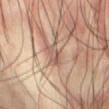This lesion was catalogued during total-body skin photography and was not selected for biopsy. The lesion is located on the abdomen. Imaged with cross-polarized lighting. The subject is a male aged 68–72. Longest diameter approximately 3 mm. A 15 mm close-up extracted from a 3D total-body photography capture.